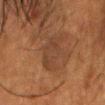notes — no biopsy performed (imaged during a skin exam) | TBP lesion metrics — a lesion color around L≈31 a*≈16 b*≈25 in CIELAB, a lesion–skin lightness drop of about 5, and a normalized border contrast of about 5; a within-lesion color-variation index near 2.5/10 and radial color variation of about 1; a nevus-likeness score of about 0/100 and a lesion-detection confidence of about 90/100 | site — the head or neck | acquisition — total-body-photography crop, ~15 mm field of view | size — ~5.5 mm (longest diameter) | patient — male, approximately 60 years of age.